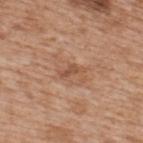{
  "biopsy_status": "not biopsied; imaged during a skin examination",
  "site": "upper back",
  "image": {
    "source": "total-body photography crop",
    "field_of_view_mm": 15
  },
  "lesion_size": {
    "long_diameter_mm_approx": 3.0
  },
  "lighting": "white-light",
  "patient": {
    "sex": "male",
    "age_approx": 65
  }
}